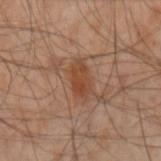notes: catalogued during a skin exam; not biopsied | diameter: about 3.5 mm | acquisition: total-body-photography crop, ~15 mm field of view | TBP lesion metrics: a border-irregularity index near 2/10 and a peripheral color-asymmetry measure near 1 | lighting: cross-polarized | anatomic site: the left arm | subject: male, aged around 50.